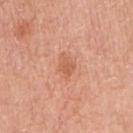biopsy_status: not biopsied; imaged during a skin examination
lesion_size:
  long_diameter_mm_approx: 3.0
automated_metrics:
  vs_skin_contrast_norm: 5.5
  border_irregularity_0_10: 3.0
  color_variation_0_10: 2.5
  peripheral_color_asymmetry: 1.0
patient:
  sex: female
  age_approx: 65
image:
  source: total-body photography crop
  field_of_view_mm: 15
site: right upper arm